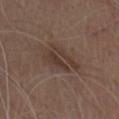Findings:
– biopsy status: no biopsy performed (imaged during a skin exam)
– tile lighting: white-light
– size: ≈5 mm
– patient: male, about 70 years old
– image: ~15 mm tile from a whole-body skin photo
– TBP lesion metrics: a lesion area of about 11 mm² and a shape eccentricity near 0.8; a lesion color around L≈37 a*≈15 b*≈23 in CIELAB and about 7 CIELAB-L* units darker than the surrounding skin; border irregularity of about 4.5 on a 0–10 scale, internal color variation of about 4 on a 0–10 scale, and peripheral color asymmetry of about 1.5; a detector confidence of about 100 out of 100 that the crop contains a lesion
– location: the chest